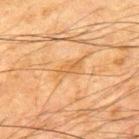Q: Is there a histopathology result?
A: no biopsy performed (imaged during a skin exam)
Q: Patient demographics?
A: male, aged 63 to 67
Q: What did automated image analysis measure?
A: an average lesion color of about L≈51 a*≈19 b*≈38 (CIELAB); border irregularity of about 4 on a 0–10 scale, a color-variation rating of about 2/10, and radial color variation of about 0.5
Q: Lesion size?
A: ~4 mm (longest diameter)
Q: What is the imaging modality?
A: 15 mm crop, total-body photography
Q: Where on the body is the lesion?
A: the upper back
Q: What lighting was used for the tile?
A: cross-polarized